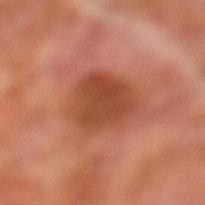A male subject, roughly 70 years of age. The lesion is located on the left lower leg. A close-up tile cropped from a whole-body skin photograph, about 15 mm across. Imaged with cross-polarized lighting. The lesion's longest dimension is about 5.5 mm.